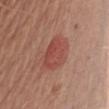Q: Is there a histopathology result?
A: no biopsy performed (imaged during a skin exam)
Q: What is the anatomic site?
A: the left upper arm
Q: How was this image acquired?
A: ~15 mm crop, total-body skin-cancer survey
Q: Who is the patient?
A: male, aged 53–57
Q: How large is the lesion?
A: about 4 mm